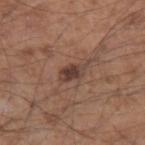Impression:
This lesion was catalogued during total-body skin photography and was not selected for biopsy.
Context:
Located on the left forearm. A 15 mm crop from a total-body photograph taken for skin-cancer surveillance. Measured at roughly 3 mm in maximum diameter. A male subject, approximately 55 years of age.